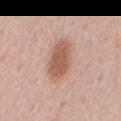The lesion was tiled from a total-body skin photograph and was not biopsied.
Imaged with white-light lighting.
Automated tile analysis of the lesion measured a lesion area of about 12 mm².
About 5.5 mm across.
A male patient, in their 70s.
Located on the mid back.
A 15 mm crop from a total-body photograph taken for skin-cancer surveillance.